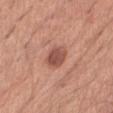No biopsy was performed on this lesion — it was imaged during a full skin examination and was not determined to be concerning. Approximately 3.5 mm at its widest. Automated image analysis of the tile measured an area of roughly 6.5 mm², a shape eccentricity near 0.65, and two-axis asymmetry of about 0.2. And it measured a lesion color around L≈51 a*≈25 b*≈28 in CIELAB, a lesion–skin lightness drop of about 12, and a normalized lesion–skin contrast near 8. The software also gave a border-irregularity index near 2/10 and a color-variation rating of about 3.5/10. The analysis additionally found a nevus-likeness score of about 55/100 and a lesion-detection confidence of about 100/100. The lesion is located on the front of the torso. A region of skin cropped from a whole-body photographic capture, roughly 15 mm wide. A male subject, in their mid-60s.